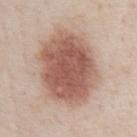Q: What kind of image is this?
A: ~15 mm crop, total-body skin-cancer survey
Q: What are the patient's age and sex?
A: male, in their 60s
Q: What is the anatomic site?
A: the chest
Q: Automated lesion metrics?
A: a footprint of about 43 mm², a shape eccentricity near 0.65, and a symmetry-axis asymmetry near 0.1; a border-irregularity index near 1.5/10, internal color variation of about 5 on a 0–10 scale, and a peripheral color-asymmetry measure near 1.5